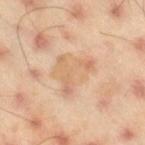Clinical impression: This lesion was catalogued during total-body skin photography and was not selected for biopsy. Image and clinical context: Measured at roughly 5 mm in maximum diameter. A roughly 15 mm field-of-view crop from a total-body skin photograph. From the right thigh. Captured under cross-polarized illumination. The patient is a male in their mid-40s.